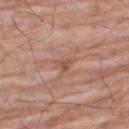Imaged during a routine full-body skin examination; the lesion was not biopsied and no histopathology is available. A lesion tile, about 15 mm wide, cut from a 3D total-body photograph. The lesion's longest dimension is about 2.5 mm. The lesion-visualizer software estimated an eccentricity of roughly 0.85 and a shape-asymmetry score of about 0.45 (0 = symmetric). The lesion is on the leg. Imaged with white-light lighting. The subject is a male about 80 years old.